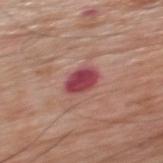<case>
<biopsy_status>not biopsied; imaged during a skin examination</biopsy_status>
<lighting>white-light</lighting>
<patient>
  <sex>male</sex>
  <age_approx>80</age_approx>
</patient>
<image>
  <source>total-body photography crop</source>
  <field_of_view_mm>15</field_of_view_mm>
</image>
<lesion_size>
  <long_diameter_mm_approx>3.5</long_diameter_mm_approx>
</lesion_size>
<automated_metrics>
  <area_mm2_approx>7.0</area_mm2_approx>
  <shape_asymmetry>0.1</shape_asymmetry>
  <border_irregularity_0_10>1.5</border_irregularity_0_10>
  <color_variation_0_10>3.0</color_variation_0_10>
  <peripheral_color_asymmetry>1.0</peripheral_color_asymmetry>
  <nevus_likeness_0_100>0</nevus_likeness_0_100>
</automated_metrics>
<site>upper back</site>
</case>